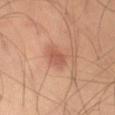{
  "biopsy_status": "not biopsied; imaged during a skin examination",
  "site": "left thigh",
  "automated_metrics": {
    "area_mm2_approx": 3.0,
    "eccentricity": 0.75,
    "shape_asymmetry": 0.25,
    "cielab_L": 53,
    "cielab_a": 25,
    "cielab_b": 30,
    "vs_skin_darker_L": 8.0,
    "vs_skin_contrast_norm": 5.5,
    "border_irregularity_0_10": 2.0,
    "color_variation_0_10": 1.5,
    "peripheral_color_asymmetry": 0.5,
    "lesion_detection_confidence_0_100": 100
  },
  "lesion_size": {
    "long_diameter_mm_approx": 2.5
  },
  "patient": {
    "sex": "male",
    "age_approx": 45
  },
  "image": {
    "source": "total-body photography crop",
    "field_of_view_mm": 15
  }
}